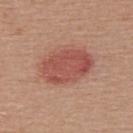No biopsy was performed on this lesion — it was imaged during a full skin examination and was not determined to be concerning.
Cropped from a total-body skin-imaging series; the visible field is about 15 mm.
The lesion is located on the upper back.
The patient is a male aged around 55.
Imaged with white-light lighting.